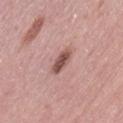The lesion is located on the lower back. About 3.5 mm across. Imaged with white-light lighting. Cropped from a total-body skin-imaging series; the visible field is about 15 mm. A female subject, approximately 55 years of age.